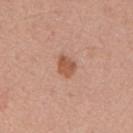{
  "site": "back",
  "image": {
    "source": "total-body photography crop",
    "field_of_view_mm": 15
  },
  "patient": {
    "sex": "male",
    "age_approx": 55
  }
}